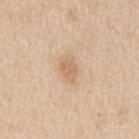{"biopsy_status": "not biopsied; imaged during a skin examination", "lighting": "white-light", "lesion_size": {"long_diameter_mm_approx": 2.5}, "site": "mid back", "image": {"source": "total-body photography crop", "field_of_view_mm": 15}, "patient": {"sex": "male", "age_approx": 60}}